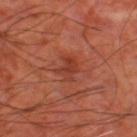Notes:
– TBP lesion metrics: a lesion area of about 5 mm², a shape eccentricity near 0.65, and a shape-asymmetry score of about 0.4 (0 = symmetric); a border-irregularity rating of about 4.5/10, a within-lesion color-variation index near 2.5/10, and a peripheral color-asymmetry measure near 0.5
– acquisition: total-body-photography crop, ~15 mm field of view
– lesion diameter: ~3 mm (longest diameter)
– anatomic site: the left thigh
– tile lighting: cross-polarized
– patient: aged 63–67